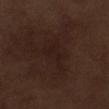biopsy status: no biopsy performed (imaged during a skin exam)